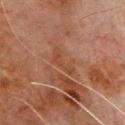notes = imaged on a skin check; not biopsied | size = about 4 mm | patient = male, roughly 80 years of age | location = the front of the torso | imaging modality = total-body-photography crop, ~15 mm field of view.